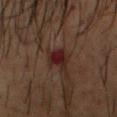Assessment: Captured during whole-body skin photography for melanoma surveillance; the lesion was not biopsied. Image and clinical context: Cropped from a whole-body photographic skin survey; the tile spans about 15 mm. The patient is a male roughly 50 years of age. Imaged with cross-polarized lighting. From the head or neck. The total-body-photography lesion software estimated a lesion color around L≈18 a*≈18 b*≈17 in CIELAB. And it measured a border-irregularity index near 2.5/10, a color-variation rating of about 4/10, and peripheral color asymmetry of about 1.5. And it measured an automated nevus-likeness rating near 0 out of 100 and lesion-presence confidence of about 100/100. The recorded lesion diameter is about 3 mm.